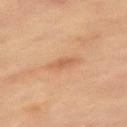biopsy_status: not biopsied; imaged during a skin examination
lesion_size:
  long_diameter_mm_approx: 3.0
image:
  source: total-body photography crop
  field_of_view_mm: 15
lighting: cross-polarized
site: left upper arm
automated_metrics:
  area_mm2_approx: 3.5
  shape_asymmetry: 0.2
  cielab_L: 51
  cielab_a: 21
  cielab_b: 32
  vs_skin_darker_L: 7.0
  vs_skin_contrast_norm: 5.0
  border_irregularity_0_10: 2.0
  peripheral_color_asymmetry: 0.5
patient:
  sex: female
  age_approx: 80